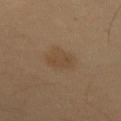Acquisition and patient details: A male patient, in their mid- to late 50s. On the mid back. The tile uses cross-polarized illumination. An algorithmic analysis of the crop reported a footprint of about 8 mm² and a shape-asymmetry score of about 0.15 (0 = symmetric). The software also gave a nevus-likeness score of about 15/100 and a lesion-detection confidence of about 100/100. About 3 mm across. A 15 mm close-up extracted from a 3D total-body photography capture.